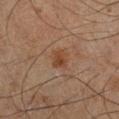Assessment: Part of a total-body skin-imaging series; this lesion was reviewed on a skin check and was not flagged for biopsy. Context: The patient is a male roughly 65 years of age. The tile uses cross-polarized illumination. A lesion tile, about 15 mm wide, cut from a 3D total-body photograph. Automated tile analysis of the lesion measured a footprint of about 4.5 mm², an outline eccentricity of about 0.5 (0 = round, 1 = elongated), and a shape-asymmetry score of about 0.25 (0 = symmetric). Longest diameter approximately 2.5 mm. On the right lower leg.